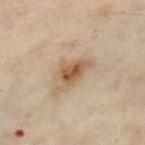A region of skin cropped from a whole-body photographic capture, roughly 15 mm wide. A female subject in their 60s. On the left thigh.